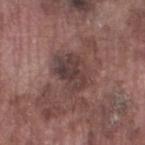Image and clinical context: This is a white-light tile. A male patient aged approximately 75. A 15 mm crop from a total-body photograph taken for skin-cancer surveillance. On the left thigh. Longest diameter approximately 4.5 mm. The lesion-visualizer software estimated an area of roughly 12 mm² and a symmetry-axis asymmetry near 0.25. The analysis additionally found a within-lesion color-variation index near 4.5/10 and peripheral color asymmetry of about 1.5.